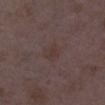follow-up — total-body-photography surveillance lesion; no biopsy | image-analysis metrics — a lesion color around L≈35 a*≈14 b*≈17 in CIELAB, roughly 4 lightness units darker than nearby skin, and a lesion-to-skin contrast of about 4.5 (normalized; higher = more distinct); a nevus-likeness score of about 0/100 and a lesion-detection confidence of about 100/100 | subject — female, in their mid- to late 30s | site — the leg | diameter — ≈2.5 mm | imaging modality — total-body-photography crop, ~15 mm field of view.